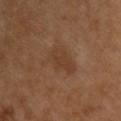Clinical impression:
Imaged during a routine full-body skin examination; the lesion was not biopsied and no histopathology is available.
Context:
From the upper back. The total-body-photography lesion software estimated a footprint of about 9 mm². It also reported a within-lesion color-variation index near 1.5/10. The software also gave a lesion-detection confidence of about 100/100. A close-up tile cropped from a whole-body skin photograph, about 15 mm across. The lesion's longest dimension is about 4.5 mm.